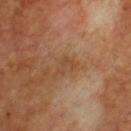Case summary:
• notes · catalogued during a skin exam; not biopsied
• body site · the front of the torso
• image · ~15 mm crop, total-body skin-cancer survey
• tile lighting · cross-polarized illumination
• subject · male, aged around 65
• lesion diameter · about 2.5 mm